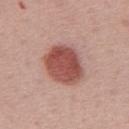Q: Was a biopsy performed?
A: imaged on a skin check; not biopsied
Q: Lesion size?
A: ≈5 mm
Q: Automated lesion metrics?
A: an automated nevus-likeness rating near 100 out of 100 and a detector confidence of about 100 out of 100 that the crop contains a lesion
Q: Where on the body is the lesion?
A: the mid back
Q: What kind of image is this?
A: total-body-photography crop, ~15 mm field of view
Q: Patient demographics?
A: male, about 55 years old